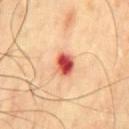Impression:
No biopsy was performed on this lesion — it was imaged during a full skin examination and was not determined to be concerning.
Context:
The recorded lesion diameter is about 3 mm. The lesion is located on the chest. A 15 mm crop from a total-body photograph taken for skin-cancer surveillance. This is a cross-polarized tile. The patient is a male aged approximately 70.